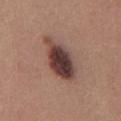Findings:
– notes: catalogued during a skin exam; not biopsied
– image-analysis metrics: a lesion area of about 16 mm² and a shape eccentricity near 0.85; a mean CIELAB color near L≈41 a*≈19 b*≈21, about 16 CIELAB-L* units darker than the surrounding skin, and a lesion-to-skin contrast of about 12.5 (normalized; higher = more distinct); a nevus-likeness score of about 70/100
– image source: ~15 mm crop, total-body skin-cancer survey
– lesion diameter: about 6 mm
– illumination: white-light
– subject: male, aged 23 to 27
– anatomic site: the front of the torso